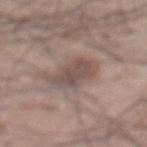Part of a total-body skin-imaging series; this lesion was reviewed on a skin check and was not flagged for biopsy.
On the mid back.
A 15 mm crop from a total-body photograph taken for skin-cancer surveillance.
The patient is a male approximately 70 years of age.